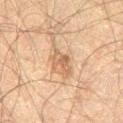A male subject aged approximately 60. Located on the leg. Cropped from a whole-body photographic skin survey; the tile spans about 15 mm. About 3 mm across.